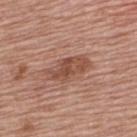Impression: Captured during whole-body skin photography for melanoma surveillance; the lesion was not biopsied. Background: This is a white-light tile. On the back. Longest diameter approximately 5 mm. A male patient aged approximately 80. A roughly 15 mm field-of-view crop from a total-body skin photograph.